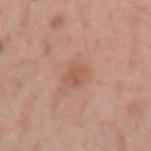A region of skin cropped from a whole-body photographic capture, roughly 15 mm wide.
From the arm.
A male patient in their 40s.
Longest diameter approximately 2.5 mm.
The total-body-photography lesion software estimated a footprint of about 4.5 mm². The analysis additionally found a color-variation rating of about 2/10 and radial color variation of about 0.5.
Imaged with white-light lighting.